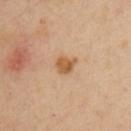Context: The tile uses cross-polarized illumination. Located on the front of the torso. A 15 mm close-up tile from a total-body photography series done for melanoma screening. A male subject aged 38–42.